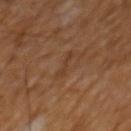notes: total-body-photography surveillance lesion; no biopsy | lighting: cross-polarized illumination | automated lesion analysis: a lesion color around L≈36 a*≈18 b*≈29 in CIELAB and a lesion–skin lightness drop of about 5; a border-irregularity rating of about 6.5/10, a within-lesion color-variation index near 0/10, and a peripheral color-asymmetry measure near 0 | imaging modality: 15 mm crop, total-body photography | subject: male, in their mid-60s | location: the mid back.